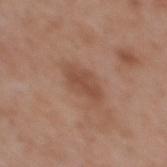Assessment: Recorded during total-body skin imaging; not selected for excision or biopsy. Acquisition and patient details: This is a white-light tile. A female patient in their mid-30s. The lesion is located on the upper back. A roughly 15 mm field-of-view crop from a total-body skin photograph.